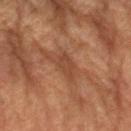{"image": {"source": "total-body photography crop", "field_of_view_mm": 15}, "patient": {"sex": "female", "age_approx": 80}, "lighting": "cross-polarized", "site": "right forearm"}